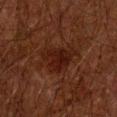• follow-up: total-body-photography surveillance lesion; no biopsy
• site: the right forearm
• image: ~15 mm tile from a whole-body skin photo
• subject: male, about 60 years old